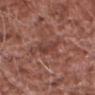Q: Was a biopsy performed?
A: no biopsy performed (imaged during a skin exam)
Q: How large is the lesion?
A: about 3 mm
Q: Where on the body is the lesion?
A: the head or neck
Q: What kind of image is this?
A: 15 mm crop, total-body photography
Q: Automated lesion metrics?
A: an eccentricity of roughly 0.8 and a shape-asymmetry score of about 0.4 (0 = symmetric); roughly 7 lightness units darker than nearby skin and a normalized lesion–skin contrast near 6; border irregularity of about 4.5 on a 0–10 scale, a color-variation rating of about 2/10, and a peripheral color-asymmetry measure near 0.5
Q: What are the patient's age and sex?
A: male, aged 73 to 77